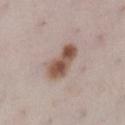<record>
  <automated_metrics>
    <area_mm2_approx>9.5</area_mm2_approx>
    <eccentricity>0.85</eccentricity>
    <shape_asymmetry>0.25</shape_asymmetry>
    <cielab_L>52</cielab_L>
    <cielab_a>18</cielab_a>
    <cielab_b>26</cielab_b>
    <vs_skin_darker_L>14.0</vs_skin_darker_L>
    <vs_skin_contrast_norm>10.5</vs_skin_contrast_norm>
    <color_variation_0_10>6.5</color_variation_0_10>
    <peripheral_color_asymmetry>2.5</peripheral_color_asymmetry>
    <nevus_likeness_0_100>85</nevus_likeness_0_100>
    <lesion_detection_confidence_0_100>100</lesion_detection_confidence_0_100>
  </automated_metrics>
  <image>
    <source>total-body photography crop</source>
    <field_of_view_mm>15</field_of_view_mm>
  </image>
  <patient>
    <sex>female</sex>
    <age_approx>30</age_approx>
  </patient>
  <lighting>white-light</lighting>
  <site>left lower leg</site>
  <lesion_size>
    <long_diameter_mm_approx>5.0</long_diameter_mm_approx>
  </lesion_size>
</record>